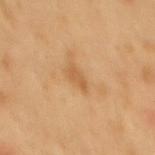Imaged during a routine full-body skin examination; the lesion was not biopsied and no histopathology is available. Cropped from a total-body skin-imaging series; the visible field is about 15 mm. A male subject, approximately 50 years of age. The lesion is on the back. This is a cross-polarized tile. An algorithmic analysis of the crop reported a lesion area of about 3.5 mm² and an eccentricity of roughly 0.8. And it measured a lesion-to-skin contrast of about 5.5 (normalized; higher = more distinct). And it measured a classifier nevus-likeness of about 0/100.